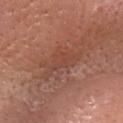Captured during whole-body skin photography for melanoma surveillance; the lesion was not biopsied.
A female subject in their mid-40s.
From the head or neck.
Imaged with white-light lighting.
Cropped from a whole-body photographic skin survey; the tile spans about 15 mm.